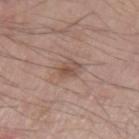image source: ~15 mm crop, total-body skin-cancer survey
diameter: ≈2.5 mm
site: the left upper arm
automated metrics: a footprint of about 4.5 mm², an eccentricity of roughly 0.55, and a shape-asymmetry score of about 0.3 (0 = symmetric); a mean CIELAB color near L≈51 a*≈18 b*≈25, about 9 CIELAB-L* units darker than the surrounding skin, and a normalized border contrast of about 6.5; border irregularity of about 2.5 on a 0–10 scale and peripheral color asymmetry of about 1.5
illumination: white-light
subject: male, aged around 70